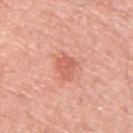The lesion was tiled from a total-body skin photograph and was not biopsied.
The patient is a male aged approximately 65.
Automated tile analysis of the lesion measured a lesion area of about 5.5 mm² and a shape eccentricity near 0.7. And it measured a mean CIELAB color near L≈62 a*≈30 b*≈32, a lesion–skin lightness drop of about 9, and a normalized border contrast of about 6. The software also gave a classifier nevus-likeness of about 15/100 and lesion-presence confidence of about 100/100.
The recorded lesion diameter is about 3 mm.
The tile uses white-light illumination.
A roughly 15 mm field-of-view crop from a total-body skin photograph.
On the upper back.